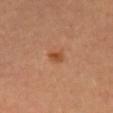follow-up: catalogued during a skin exam; not biopsied
automated metrics: a footprint of about 3 mm² and a shape eccentricity near 0.6; a classifier nevus-likeness of about 85/100
subject: male, in their 60s
image: ~15 mm crop, total-body skin-cancer survey
lesion diameter: about 2 mm
tile lighting: cross-polarized illumination
location: the mid back